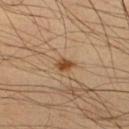The lesion was tiled from a total-body skin photograph and was not biopsied.
A male patient roughly 45 years of age.
The lesion's longest dimension is about 2.5 mm.
Cropped from a total-body skin-imaging series; the visible field is about 15 mm.
From the right lower leg.
Imaged with cross-polarized lighting.